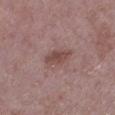Notes:
- workup — catalogued during a skin exam; not biopsied
- patient — male, about 70 years old
- TBP lesion metrics — a normalized lesion–skin contrast near 7; internal color variation of about 2 on a 0–10 scale and a peripheral color-asymmetry measure near 0.5; lesion-presence confidence of about 100/100
- illumination — white-light illumination
- imaging modality — total-body-photography crop, ~15 mm field of view
- site — the left lower leg
- size — about 4 mm